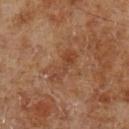Clinical impression: Recorded during total-body skin imaging; not selected for excision or biopsy. Context: Located on the left lower leg. A male patient aged 68–72. The lesion's longest dimension is about 4.5 mm. An algorithmic analysis of the crop reported a footprint of about 6 mm². It also reported a border-irregularity index near 4.5/10, a color-variation rating of about 3/10, and radial color variation of about 1. The software also gave an automated nevus-likeness rating near 0 out of 100 and lesion-presence confidence of about 100/100. This image is a 15 mm lesion crop taken from a total-body photograph. The tile uses cross-polarized illumination.